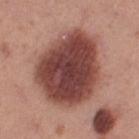anatomic site: the right thigh
image source: ~15 mm tile from a whole-body skin photo
lighting: white-light
automated lesion analysis: an area of roughly 49 mm², an eccentricity of roughly 0.6, and a shape-asymmetry score of about 0.15 (0 = symmetric); a mean CIELAB color near L≈44 a*≈24 b*≈24 and a normalized border contrast of about 13
subject: female, aged approximately 40
size: ~9 mm (longest diameter)